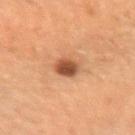Recorded during total-body skin imaging; not selected for excision or biopsy.
Captured under cross-polarized illumination.
The lesion's longest dimension is about 2.5 mm.
From the arm.
A roughly 15 mm field-of-view crop from a total-body skin photograph.
Automated tile analysis of the lesion measured an average lesion color of about L≈43 a*≈21 b*≈31 (CIELAB) and roughly 13 lightness units darker than nearby skin.
A female subject approximately 55 years of age.